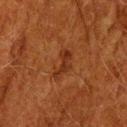Imaged during a routine full-body skin examination; the lesion was not biopsied and no histopathology is available. A male subject aged 78 to 82. A lesion tile, about 15 mm wide, cut from a 3D total-body photograph. The lesion is located on the head or neck.